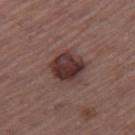Assessment: Part of a total-body skin-imaging series; this lesion was reviewed on a skin check and was not flagged for biopsy. Acquisition and patient details: The lesion-visualizer software estimated an area of roughly 12 mm², an eccentricity of roughly 0.45, and a shape-asymmetry score of about 0.2 (0 = symmetric). Measured at roughly 4 mm in maximum diameter. The subject is a male aged approximately 65. On the right thigh. The tile uses white-light illumination. A 15 mm close-up tile from a total-body photography series done for melanoma screening.